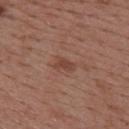Clinical impression:
Captured during whole-body skin photography for melanoma surveillance; the lesion was not biopsied.
Background:
Located on the upper back. The tile uses white-light illumination. A female subject, aged approximately 50. This image is a 15 mm lesion crop taken from a total-body photograph.